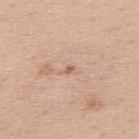The patient is a male about 40 years old. A roughly 15 mm field-of-view crop from a total-body skin photograph. From the back.